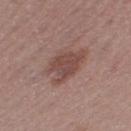| key | value |
|---|---|
| biopsy status | no biopsy performed (imaged during a skin exam) |
| subject | female, approximately 55 years of age |
| body site | the left thigh |
| acquisition | ~15 mm tile from a whole-body skin photo |